The lesion is on the right upper arm. The patient is a female aged 38–42. Cropped from a whole-body photographic skin survey; the tile spans about 15 mm: 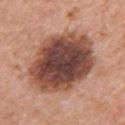Diagnosis:
Histopathologically confirmed as a dysplastic (Clark) nevus.